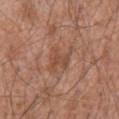{
  "biopsy_status": "not biopsied; imaged during a skin examination",
  "automated_metrics": {
    "cielab_L": 49,
    "cielab_a": 21,
    "cielab_b": 30,
    "vs_skin_darker_L": 7.0,
    "vs_skin_contrast_norm": 5.5,
    "color_variation_0_10": 2.0,
    "lesion_detection_confidence_0_100": 95
  },
  "site": "back",
  "lesion_size": {
    "long_diameter_mm_approx": 4.5
  },
  "image": {
    "source": "total-body photography crop",
    "field_of_view_mm": 15
  },
  "lighting": "white-light",
  "patient": {
    "sex": "male",
    "age_approx": 60
  }
}